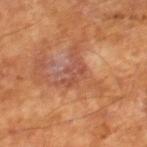The lesion was photographed on a routine skin check and not biopsied; there is no pathology result. The tile uses cross-polarized illumination. The subject is a male in their mid- to late 60s. About 3 mm across. This image is a 15 mm lesion crop taken from a total-body photograph. Automated image analysis of the tile measured a mean CIELAB color near L≈49 a*≈27 b*≈31 and a normalized border contrast of about 5.5. It also reported a color-variation rating of about 1/10 and a peripheral color-asymmetry measure near 0.5. The software also gave a classifier nevus-likeness of about 0/100.